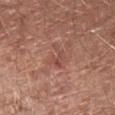* notes: catalogued during a skin exam; not biopsied
* body site: the right forearm
* illumination: white-light
* diameter: ~3 mm (longest diameter)
* patient: female, in their 60s
* imaging modality: ~15 mm tile from a whole-body skin photo